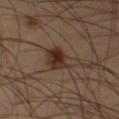From the leg. A male subject, about 65 years old. The tile uses cross-polarized illumination. A 15 mm close-up extracted from a 3D total-body photography capture. The lesion-visualizer software estimated a footprint of about 9.5 mm² and an eccentricity of roughly 0.8. The analysis additionally found a mean CIELAB color near L≈31 a*≈15 b*≈24, a lesion–skin lightness drop of about 9, and a lesion-to-skin contrast of about 9 (normalized; higher = more distinct). About 5 mm across.